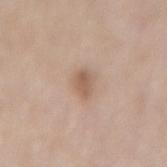Captured during whole-body skin photography for melanoma surveillance; the lesion was not biopsied. A female subject aged approximately 60. The lesion-visualizer software estimated a footprint of about 4 mm² and a symmetry-axis asymmetry near 0.2. And it measured an average lesion color of about L≈58 a*≈17 b*≈28 (CIELAB), about 10 CIELAB-L* units darker than the surrounding skin, and a lesion-to-skin contrast of about 7 (normalized; higher = more distinct). The analysis additionally found an automated nevus-likeness rating near 75 out of 100. A 15 mm close-up extracted from a 3D total-body photography capture. From the lower back. The tile uses white-light illumination.